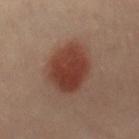biopsy_status: not biopsied; imaged during a skin examination
lesion_size:
  long_diameter_mm_approx: 5.5
lighting: cross-polarized
site: left leg
image:
  source: total-body photography crop
  field_of_view_mm: 15
patient:
  sex: female
  age_approx: 40